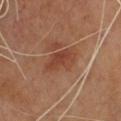Findings:
• follow-up: imaged on a skin check; not biopsied
• lesion diameter: ≈4.5 mm
• subject: male, aged approximately 70
• image source: total-body-photography crop, ~15 mm field of view
• illumination: cross-polarized illumination
• location: the chest